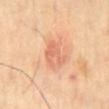{
  "image": {
    "source": "total-body photography crop",
    "field_of_view_mm": 15
  },
  "site": "back",
  "automated_metrics": {
    "eccentricity": 0.55,
    "nevus_likeness_0_100": 5,
    "lesion_detection_confidence_0_100": 100
  },
  "lighting": "cross-polarized",
  "patient": {
    "sex": "male",
    "age_approx": 65
  }
}